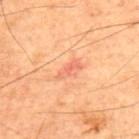A male patient, approximately 60 years of age. Measured at roughly 3 mm in maximum diameter. The lesion is on the upper back. A roughly 15 mm field-of-view crop from a total-body skin photograph.